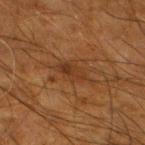<tbp_lesion>
  <biopsy_status>not biopsied; imaged during a skin examination</biopsy_status>
  <lighting>cross-polarized</lighting>
  <patient>
    <sex>male</sex>
    <age_approx>65</age_approx>
  </patient>
  <image>
    <source>total-body photography crop</source>
    <field_of_view_mm>15</field_of_view_mm>
  </image>
  <site>left lower leg</site>
  <lesion_size>
    <long_diameter_mm_approx>4.0</long_diameter_mm_approx>
  </lesion_size>
</tbp_lesion>